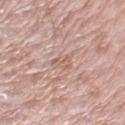The lesion was photographed on a routine skin check and not biopsied; there is no pathology result. A region of skin cropped from a whole-body photographic capture, roughly 15 mm wide. The total-body-photography lesion software estimated an outline eccentricity of about 0.7 (0 = round, 1 = elongated) and two-axis asymmetry of about 0.45. The analysis additionally found a mean CIELAB color near L≈61 a*≈19 b*≈26, roughly 7 lightness units darker than nearby skin, and a normalized border contrast of about 4.5. It also reported internal color variation of about 2 on a 0–10 scale and a peripheral color-asymmetry measure near 1. A male subject, aged approximately 75. Captured under white-light illumination. Located on the left upper arm. Longest diameter approximately 3 mm.